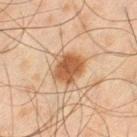– site — the leg
– lighting — cross-polarized illumination
– image source — ~15 mm tile from a whole-body skin photo
– size — ~3.5 mm (longest diameter)
– patient — male, roughly 45 years of age